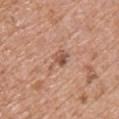Assessment: Imaged during a routine full-body skin examination; the lesion was not biopsied and no histopathology is available. Acquisition and patient details: Automated image analysis of the tile measured an area of roughly 4.5 mm², a shape eccentricity near 0.85, and a shape-asymmetry score of about 0.5 (0 = symmetric). It also reported a classifier nevus-likeness of about 5/100. The patient is a female approximately 40 years of age. On the chest. This is a white-light tile. Cropped from a whole-body photographic skin survey; the tile spans about 15 mm.